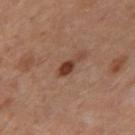Background:
The recorded lesion diameter is about 2.5 mm. Captured under cross-polarized illumination. The lesion is on the left thigh. Cropped from a total-body skin-imaging series; the visible field is about 15 mm. A female patient aged approximately 50.